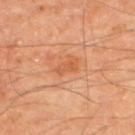– follow-up · no biopsy performed (imaged during a skin exam)
– acquisition · 15 mm crop, total-body photography
– patient · male, aged approximately 65
– lesion diameter · ≈2.5 mm
– automated lesion analysis · a border-irregularity index near 3/10, internal color variation of about 2 on a 0–10 scale, and a peripheral color-asymmetry measure near 0.5
– tile lighting · cross-polarized illumination
– location · the upper back